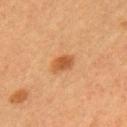Context:
The recorded lesion diameter is about 3 mm. A female subject, aged approximately 55. Cropped from a total-body skin-imaging series; the visible field is about 15 mm. From the left upper arm. Imaged with cross-polarized lighting.